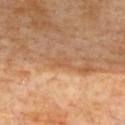The lesion was photographed on a routine skin check and not biopsied; there is no pathology result. The lesion-visualizer software estimated a lesion–skin lightness drop of about 6 and a lesion-to-skin contrast of about 4.5 (normalized; higher = more distinct). The analysis additionally found a color-variation rating of about 0/10 and a peripheral color-asymmetry measure near 0. Imaged with cross-polarized lighting. Cropped from a total-body skin-imaging series; the visible field is about 15 mm. On the upper back. A female subject in their mid-60s. The lesion's longest dimension is about 2 mm.